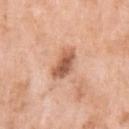Recorded during total-body skin imaging; not selected for excision or biopsy.
A female subject roughly 70 years of age.
Measured at roughly 4 mm in maximum diameter.
Automated tile analysis of the lesion measured a lesion area of about 7 mm², a shape eccentricity near 0.75, and two-axis asymmetry of about 0.3. And it measured a normalized lesion–skin contrast near 9. It also reported a border-irregularity index near 3/10, internal color variation of about 4 on a 0–10 scale, and radial color variation of about 1.5. The software also gave an automated nevus-likeness rating near 35 out of 100 and a detector confidence of about 100 out of 100 that the crop contains a lesion.
The lesion is located on the left upper arm.
Imaged with white-light lighting.
A lesion tile, about 15 mm wide, cut from a 3D total-body photograph.